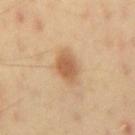This lesion was catalogued during total-body skin photography and was not selected for biopsy.
About 3 mm across.
Captured under cross-polarized illumination.
Located on the mid back.
The patient is a male approximately 40 years of age.
A close-up tile cropped from a whole-body skin photograph, about 15 mm across.